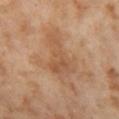Imaged during a routine full-body skin examination; the lesion was not biopsied and no histopathology is available. A region of skin cropped from a whole-body photographic capture, roughly 15 mm wide. The recorded lesion diameter is about 5.5 mm. Automated tile analysis of the lesion measured an eccentricity of roughly 0.8. The patient is a female roughly 55 years of age. The tile uses cross-polarized illumination. Located on the left thigh.